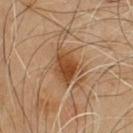<lesion>
  <lighting>cross-polarized</lighting>
  <patient>
    <sex>male</sex>
    <age_approx>55</age_approx>
  </patient>
  <lesion_size>
    <long_diameter_mm_approx>4.5</long_diameter_mm_approx>
  </lesion_size>
  <automated_metrics>
    <color_variation_0_10>5.0</color_variation_0_10>
    <peripheral_color_asymmetry>1.5</peripheral_color_asymmetry>
  </automated_metrics>
  <image>
    <source>total-body photography crop</source>
    <field_of_view_mm>15</field_of_view_mm>
  </image>
  <site>chest</site>
</lesion>